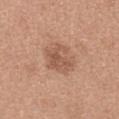{
  "biopsy_status": "not biopsied; imaged during a skin examination",
  "image": {
    "source": "total-body photography crop",
    "field_of_view_mm": 15
  },
  "site": "back",
  "lesion_size": {
    "long_diameter_mm_approx": 4.5
  },
  "patient": {
    "sex": "female",
    "age_approx": 30
  },
  "lighting": "white-light"
}